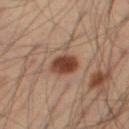Part of a total-body skin-imaging series; this lesion was reviewed on a skin check and was not flagged for biopsy. The lesion is on the right thigh. A 15 mm crop from a total-body photograph taken for skin-cancer surveillance. The patient is a male aged around 55.